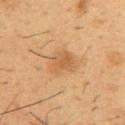{"biopsy_status": "not biopsied; imaged during a skin examination", "site": "front of the torso", "image": {"source": "total-body photography crop", "field_of_view_mm": 15}, "patient": {"sex": "male", "age_approx": 55}, "lesion_size": {"long_diameter_mm_approx": 4.5}}